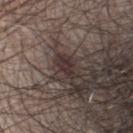Findings:
• biopsy status: imaged on a skin check; not biopsied
• patient: male, aged approximately 50
• image: ~15 mm crop, total-body skin-cancer survey
• lesion diameter: ~3 mm (longest diameter)
• body site: the head or neck